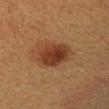{
  "biopsy_status": "not biopsied; imaged during a skin examination",
  "patient": {
    "sex": "female",
    "age_approx": 40
  },
  "image": {
    "source": "total-body photography crop",
    "field_of_view_mm": 15
  },
  "site": "left forearm"
}